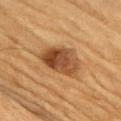follow-up — total-body-photography surveillance lesion; no biopsy
image-analysis metrics — a lesion area of about 15 mm²; a mean CIELAB color near L≈42 a*≈21 b*≈34, roughly 13 lightness units darker than nearby skin, and a normalized lesion–skin contrast near 10; a border-irregularity index near 2.5/10, a color-variation rating of about 7/10, and a peripheral color-asymmetry measure near 2.5
image source — total-body-photography crop, ~15 mm field of view
subject — male, approximately 55 years of age
location — the chest
illumination — cross-polarized illumination
size — ~5.5 mm (longest diameter)